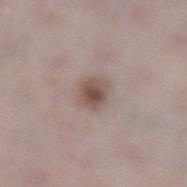| feature | finding |
|---|---|
| follow-up | catalogued during a skin exam; not biopsied |
| site | the left lower leg |
| image source | ~15 mm tile from a whole-body skin photo |
| patient | female, about 50 years old |
| image-analysis metrics | an area of roughly 6 mm², a shape eccentricity near 0.4, and a symmetry-axis asymmetry near 0.2; a lesion color around L≈51 a*≈16 b*≈21 in CIELAB and roughly 11 lightness units darker than nearby skin; a classifier nevus-likeness of about 90/100 |
| illumination | white-light illumination |
| size | about 2.5 mm |